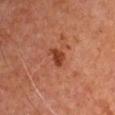This lesion was catalogued during total-body skin photography and was not selected for biopsy. The total-body-photography lesion software estimated an area of roughly 3 mm², a shape eccentricity near 0.8, and two-axis asymmetry of about 0.4. Located on the left upper arm. A male patient, about 65 years old. Cropped from a whole-body photographic skin survey; the tile spans about 15 mm. Imaged with cross-polarized lighting.